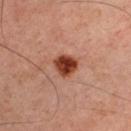biopsy_status: not biopsied; imaged during a skin examination
patient:
  sex: male
  age_approx: 45
image:
  source: total-body photography crop
  field_of_view_mm: 15
lighting: cross-polarized
lesion_size:
  long_diameter_mm_approx: 3.0
automated_metrics:
  cielab_L: 39
  cielab_a: 27
  cielab_b: 31
  border_irregularity_0_10: 2.0
  color_variation_0_10: 5.0
  peripheral_color_asymmetry: 1.5
  nevus_likeness_0_100: 100
site: arm